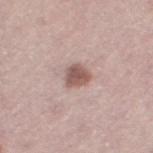Context:
The total-body-photography lesion software estimated an average lesion color of about L≈55 a*≈20 b*≈22 (CIELAB), roughly 13 lightness units darker than nearby skin, and a normalized border contrast of about 9. It also reported a nevus-likeness score of about 80/100 and a lesion-detection confidence of about 100/100. A 15 mm close-up tile from a total-body photography series done for melanoma screening. Located on the left thigh. A female patient, roughly 40 years of age. Longest diameter approximately 2.5 mm.